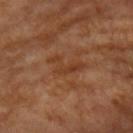The lesion was tiled from a total-body skin photograph and was not biopsied.
A male patient, aged approximately 55.
A roughly 15 mm field-of-view crop from a total-body skin photograph.
The total-body-photography lesion software estimated a lesion color around L≈38 a*≈22 b*≈33 in CIELAB, about 6 CIELAB-L* units darker than the surrounding skin, and a normalized border contrast of about 6. And it measured border irregularity of about 7.5 on a 0–10 scale, internal color variation of about 2 on a 0–10 scale, and peripheral color asymmetry of about 0.5.
This is a cross-polarized tile.
On the left upper arm.
Longest diameter approximately 4.5 mm.